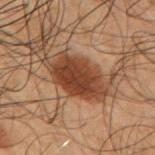Assessment:
Recorded during total-body skin imaging; not selected for excision or biopsy.
Acquisition and patient details:
The recorded lesion diameter is about 5.5 mm. The tile uses cross-polarized illumination. A roughly 15 mm field-of-view crop from a total-body skin photograph. A male subject about 50 years old. From the arm.